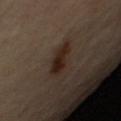Case summary:
– notes: total-body-photography surveillance lesion; no biopsy
– body site: the right upper arm
– size: ~4.5 mm (longest diameter)
– lighting: cross-polarized
– image-analysis metrics: a mean CIELAB color near L≈22 a*≈13 b*≈21, about 9 CIELAB-L* units darker than the surrounding skin, and a lesion-to-skin contrast of about 10.5 (normalized; higher = more distinct)
– acquisition: 15 mm crop, total-body photography
– patient: female, aged 63 to 67